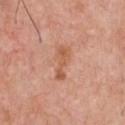The recorded lesion diameter is about 4 mm.
A roughly 15 mm field-of-view crop from a total-body skin photograph.
The patient is a male approximately 60 years of age.
From the front of the torso.
Captured under white-light illumination.